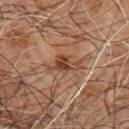This lesion was catalogued during total-body skin photography and was not selected for biopsy. A male patient, about 60 years old. Captured under cross-polarized illumination. A close-up tile cropped from a whole-body skin photograph, about 15 mm across. On the chest. The lesion-visualizer software estimated a border-irregularity index near 5.5/10, a within-lesion color-variation index near 3/10, and radial color variation of about 1. The software also gave a classifier nevus-likeness of about 5/100.